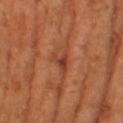<lesion>
<image>
  <source>total-body photography crop</source>
  <field_of_view_mm>15</field_of_view_mm>
</image>
<lighting>cross-polarized</lighting>
<patient>
  <sex>female</sex>
  <age_approx>55</age_approx>
</patient>
<site>right lower leg</site>
<automated_metrics>
  <area_mm2_approx>3.5</area_mm2_approx>
  <eccentricity>0.6</eccentricity>
  <shape_asymmetry>0.6</shape_asymmetry>
  <cielab_L>43</cielab_L>
  <cielab_a>29</cielab_a>
  <cielab_b>36</cielab_b>
  <vs_skin_darker_L>9.0</vs_skin_darker_L>
  <vs_skin_contrast_norm>7.0</vs_skin_contrast_norm>
  <nevus_likeness_0_100>0</nevus_likeness_0_100>
</automated_metrics>
</lesion>